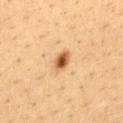Findings:
• follow-up · catalogued during a skin exam; not biopsied
• body site · the upper back
• imaging modality · ~15 mm crop, total-body skin-cancer survey
• patient · female, aged 38 to 42